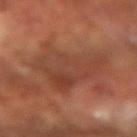No biopsy was performed on this lesion — it was imaged during a full skin examination and was not determined to be concerning. A 15 mm crop from a total-body photograph taken for skin-cancer surveillance. This is a cross-polarized tile. The lesion is on the left forearm. The subject is a male aged around 65. An algorithmic analysis of the crop reported a mean CIELAB color near L≈41 a*≈23 b*≈29, roughly 7 lightness units darker than nearby skin, and a normalized border contrast of about 6. And it measured a detector confidence of about 95 out of 100 that the crop contains a lesion.